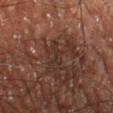| field | value |
|---|---|
| notes | catalogued during a skin exam; not biopsied |
| size | ≈1.5 mm |
| subject | male, aged around 60 |
| location | the left thigh |
| image | 15 mm crop, total-body photography |
| illumination | cross-polarized |